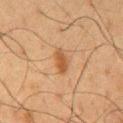biopsy_status: not biopsied; imaged during a skin examination
site: mid back
lesion_size:
  long_diameter_mm_approx: 3.0
patient:
  sex: male
  age_approx: 60
lighting: cross-polarized
image:
  source: total-body photography crop
  field_of_view_mm: 15
automated_metrics:
  cielab_L: 42
  cielab_a: 19
  cielab_b: 32
  vs_skin_darker_L: 9.0
  vs_skin_contrast_norm: 7.5
  color_variation_0_10: 1.5
  peripheral_color_asymmetry: 0.5
  nevus_likeness_0_100: 90
  lesion_detection_confidence_0_100: 100